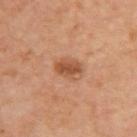Clinical impression: This lesion was catalogued during total-body skin photography and was not selected for biopsy. Acquisition and patient details: Located on the upper back. A male patient approximately 65 years of age. This is a cross-polarized tile. The recorded lesion diameter is about 3.5 mm. This image is a 15 mm lesion crop taken from a total-body photograph.